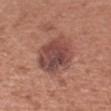Clinical impression:
Captured during whole-body skin photography for melanoma surveillance; the lesion was not biopsied.
Image and clinical context:
This image is a 15 mm lesion crop taken from a total-body photograph. Imaged with white-light lighting. An algorithmic analysis of the crop reported an area of roughly 18 mm², a shape eccentricity near 0.6, and two-axis asymmetry of about 0.15. And it measured a border-irregularity index near 2/10, internal color variation of about 6.5 on a 0–10 scale, and peripheral color asymmetry of about 2. And it measured lesion-presence confidence of about 100/100. From the right upper arm. The lesion's longest dimension is about 5.5 mm. A female subject aged around 40.